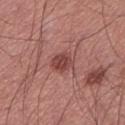This is a white-light tile. A male subject, roughly 60 years of age. Cropped from a total-body skin-imaging series; the visible field is about 15 mm. Approximately 3 mm at its widest. The lesion is on the left lower leg.